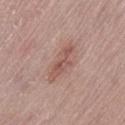Imaged during a routine full-body skin examination; the lesion was not biopsied and no histopathology is available.